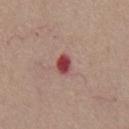Q: Is there a histopathology result?
A: total-body-photography surveillance lesion; no biopsy
Q: How was this image acquired?
A: ~15 mm tile from a whole-body skin photo
Q: Lesion location?
A: the chest
Q: What are the patient's age and sex?
A: male, aged around 55
Q: What did automated image analysis measure?
A: a mean CIELAB color near L≈45 a*≈32 b*≈23, roughly 16 lightness units darker than nearby skin, and a normalized lesion–skin contrast near 11.5; a nevus-likeness score of about 0/100
Q: What is the lesion's diameter?
A: ≈2.5 mm